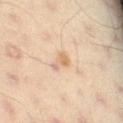The lesion was photographed on a routine skin check and not biopsied; there is no pathology result. A male subject, approximately 55 years of age. Located on the left thigh. A 15 mm close-up extracted from a 3D total-body photography capture. Longest diameter approximately 3 mm. The tile uses cross-polarized illumination.